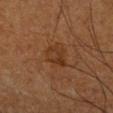The lesion was photographed on a routine skin check and not biopsied; there is no pathology result.
On the right forearm.
The recorded lesion diameter is about 3 mm.
A region of skin cropped from a whole-body photographic capture, roughly 15 mm wide.
A male subject aged approximately 55.